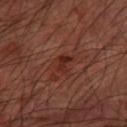Imaged during a routine full-body skin examination; the lesion was not biopsied and no histopathology is available. The tile uses cross-polarized illumination. Measured at roughly 2.5 mm in maximum diameter. The subject is a male about 65 years old. Automated tile analysis of the lesion measured a shape eccentricity near 0.7 and two-axis asymmetry of about 0.25. It also reported border irregularity of about 3 on a 0–10 scale and a color-variation rating of about 3/10. And it measured a classifier nevus-likeness of about 5/100 and a detector confidence of about 100 out of 100 that the crop contains a lesion. The lesion is located on the left forearm. A 15 mm close-up extracted from a 3D total-body photography capture.